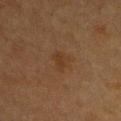Findings:
– notes · no biopsy performed (imaged during a skin exam)
– automated metrics · a lesion area of about 3.5 mm² and a symmetry-axis asymmetry near 0.35
– lesion diameter · ≈2.5 mm
– subject · female, approximately 40 years of age
– image source · 15 mm crop, total-body photography
– location · the chest